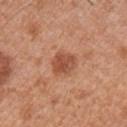From the front of the torso.
A lesion tile, about 15 mm wide, cut from a 3D total-body photograph.
A male patient, in their 30s.
The lesion's longest dimension is about 3 mm.
The tile uses white-light illumination.